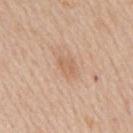Q: Is there a histopathology result?
A: catalogued during a skin exam; not biopsied
Q: What did automated image analysis measure?
A: a mean CIELAB color near L≈62 a*≈20 b*≈32 and a normalized border contrast of about 5
Q: What lighting was used for the tile?
A: white-light illumination
Q: How was this image acquired?
A: ~15 mm crop, total-body skin-cancer survey
Q: Patient demographics?
A: male, aged 58–62
Q: What is the anatomic site?
A: the mid back
Q: Lesion size?
A: ~2.5 mm (longest diameter)